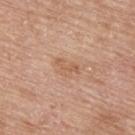* workup · no biopsy performed (imaged during a skin exam)
* site · the upper back
* patient · male, approximately 65 years of age
* image · total-body-photography crop, ~15 mm field of view